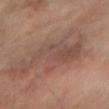| field | value |
|---|---|
| notes | no biopsy performed (imaged during a skin exam) |
| image | total-body-photography crop, ~15 mm field of view |
| subject | female, about 55 years old |
| lesion diameter | ≈8 mm |
| anatomic site | the right forearm |
| illumination | cross-polarized |